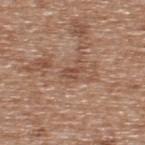| field | value |
|---|---|
| follow-up | total-body-photography surveillance lesion; no biopsy |
| size | ~2.5 mm (longest diameter) |
| subject | male, aged 63–67 |
| tile lighting | white-light illumination |
| location | the upper back |
| TBP lesion metrics | a footprint of about 2.5 mm² and a symmetry-axis asymmetry near 0.4; an automated nevus-likeness rating near 0 out of 100 and a lesion-detection confidence of about 55/100 |
| acquisition | total-body-photography crop, ~15 mm field of view |